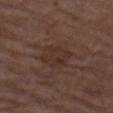The lesion was tiled from a total-body skin photograph and was not biopsied. This image is a 15 mm lesion crop taken from a total-body photograph. A male patient approximately 75 years of age. Located on the left thigh. The recorded lesion diameter is about 4 mm. An algorithmic analysis of the crop reported a lesion-detection confidence of about 100/100.